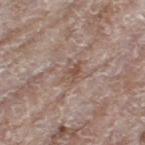acquisition = ~15 mm tile from a whole-body skin photo
subject = female, aged 73 to 77
automated metrics = a lesion area of about 3.5 mm²; a lesion-detection confidence of about 100/100
anatomic site = the left thigh
lighting = white-light